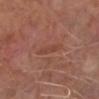notes: imaged on a skin check; not biopsied | imaging modality: ~15 mm tile from a whole-body skin photo | tile lighting: white-light | location: the right lower leg | lesion size: ≈4 mm | subject: male, aged 63–67.